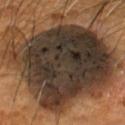Imaged during a routine full-body skin examination; the lesion was not biopsied and no histopathology is available. Measured at roughly 13.5 mm in maximum diameter. On the head or neck. The tile uses cross-polarized illumination. A male patient aged approximately 55. A roughly 15 mm field-of-view crop from a total-body skin photograph.